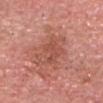Captured during whole-body skin photography for melanoma surveillance; the lesion was not biopsied.
The tile uses white-light illumination.
A male subject in their mid-60s.
The lesion is on the head or neck.
Cropped from a total-body skin-imaging series; the visible field is about 15 mm.
Approximately 5.5 mm at its widest.